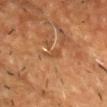Impression: The lesion was photographed on a routine skin check and not biopsied; there is no pathology result. Image and clinical context: A 15 mm close-up extracted from a 3D total-body photography capture. Measured at roughly 2.5 mm in maximum diameter. The total-body-photography lesion software estimated an area of roughly 2.5 mm² and a shape-asymmetry score of about 0.45 (0 = symmetric). And it measured a lesion color around L≈45 a*≈22 b*≈36 in CIELAB and a normalized border contrast of about 5. A male subject, aged 58 to 62. The lesion is located on the head or neck.